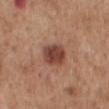Captured during whole-body skin photography for melanoma surveillance; the lesion was not biopsied. A close-up tile cropped from a whole-body skin photograph, about 15 mm across. Captured under white-light illumination. Approximately 3.5 mm at its widest. A male subject, in their 60s. The lesion is on the back. The lesion-visualizer software estimated a lesion color around L≈44 a*≈22 b*≈27 in CIELAB, a lesion–skin lightness drop of about 13, and a lesion-to-skin contrast of about 10 (normalized; higher = more distinct). The software also gave border irregularity of about 1 on a 0–10 scale and peripheral color asymmetry of about 1.